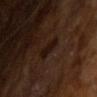The lesion was photographed on a routine skin check and not biopsied; there is no pathology result. Approximately 3 mm at its widest. The subject is a male aged approximately 65. A roughly 15 mm field-of-view crop from a total-body skin photograph. The tile uses cross-polarized illumination. The lesion-visualizer software estimated a lesion area of about 4.5 mm² and a shape-asymmetry score of about 0.3 (0 = symmetric). The analysis additionally found a border-irregularity index near 3/10, a within-lesion color-variation index near 1.5/10, and a peripheral color-asymmetry measure near 0.5. The software also gave a nevus-likeness score of about 85/100 and a lesion-detection confidence of about 100/100.